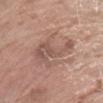A female patient aged 73–77.
A close-up tile cropped from a whole-body skin photograph, about 15 mm across.
From the right forearm.
Automated image analysis of the tile measured a footprint of about 16 mm², an eccentricity of roughly 0.8, and a shape-asymmetry score of about 0.45 (0 = symmetric). It also reported a mean CIELAB color near L≈54 a*≈19 b*≈25 and a lesion–skin lightness drop of about 9. It also reported a color-variation rating of about 3.5/10 and peripheral color asymmetry of about 1. The software also gave a lesion-detection confidence of about 100/100.
The tile uses white-light illumination.